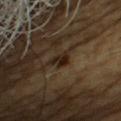workup = total-body-photography surveillance lesion; no biopsy
site = the chest
image = ~15 mm crop, total-body skin-cancer survey
illumination = cross-polarized
subject = male, approximately 60 years of age
lesion diameter = ≈2.5 mm